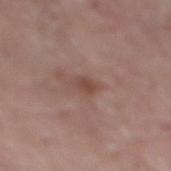The lesion was photographed on a routine skin check and not biopsied; there is no pathology result. A lesion tile, about 15 mm wide, cut from a 3D total-body photograph. From the right lower leg. A female subject, in their mid-80s or older.